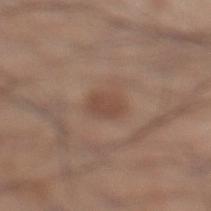The lesion was tiled from a total-body skin photograph and was not biopsied.